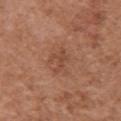biopsy status: imaged on a skin check; not biopsied
subject: female, aged 63–67
imaging modality: total-body-photography crop, ~15 mm field of view
illumination: white-light illumination
location: the left arm
diameter: about 3 mm